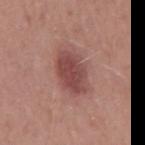<tbp_lesion>
  <biopsy_status>not biopsied; imaged during a skin examination</biopsy_status>
  <patient>
    <sex>male</sex>
    <age_approx>50</age_approx>
  </patient>
  <site>mid back</site>
  <image>
    <source>total-body photography crop</source>
    <field_of_view_mm>15</field_of_view_mm>
  </image>
</tbp_lesion>